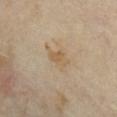Clinical impression: Captured during whole-body skin photography for melanoma surveillance; the lesion was not biopsied.